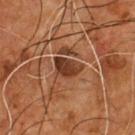Part of a total-body skin-imaging series; this lesion was reviewed on a skin check and was not flagged for biopsy.
From the chest.
This is a cross-polarized tile.
A close-up tile cropped from a whole-body skin photograph, about 15 mm across.
A male subject approximately 55 years of age.
Measured at roughly 3.5 mm in maximum diameter.